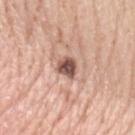– body site · the left upper arm
– illumination · white-light
– image source · ~15 mm tile from a whole-body skin photo
– patient · female, aged around 40